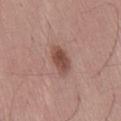Assessment:
Imaged during a routine full-body skin examination; the lesion was not biopsied and no histopathology is available.
Background:
Captured under white-light illumination. The recorded lesion diameter is about 4 mm. Cropped from a whole-body photographic skin survey; the tile spans about 15 mm. The patient is a male aged approximately 55. Located on the back.